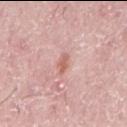The lesion was tiled from a total-body skin photograph and was not biopsied. Located on the back. Longest diameter approximately 2.5 mm. A male patient aged 73 to 77. The lesion-visualizer software estimated a mean CIELAB color near L≈62 a*≈24 b*≈27, a lesion–skin lightness drop of about 10, and a lesion-to-skin contrast of about 7 (normalized; higher = more distinct). The software also gave border irregularity of about 2.5 on a 0–10 scale and a color-variation rating of about 1/10. And it measured a nevus-likeness score of about 0/100 and a lesion-detection confidence of about 100/100. This is a white-light tile. A roughly 15 mm field-of-view crop from a total-body skin photograph.